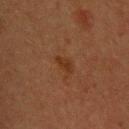biopsy status=total-body-photography surveillance lesion; no biopsy | patient=male, roughly 40 years of age | body site=the upper back | automated lesion analysis=border irregularity of about 3 on a 0–10 scale, internal color variation of about 0.5 on a 0–10 scale, and peripheral color asymmetry of about 0; a lesion-detection confidence of about 100/100 | lesion diameter=~2.5 mm (longest diameter) | acquisition=~15 mm tile from a whole-body skin photo.